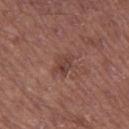Impression:
Part of a total-body skin-imaging series; this lesion was reviewed on a skin check and was not flagged for biopsy.
Background:
About 2.5 mm across. A male patient, about 55 years old. Imaged with white-light lighting. From the left thigh. Cropped from a total-body skin-imaging series; the visible field is about 15 mm.